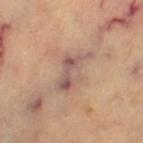{
  "biopsy_status": "not biopsied; imaged during a skin examination",
  "patient": {
    "sex": "female",
    "age_approx": 70
  },
  "lighting": "cross-polarized",
  "automated_metrics": {
    "area_mm2_approx": 10.0,
    "eccentricity": 0.85,
    "color_variation_0_10": 7.0,
    "peripheral_color_asymmetry": 2.0,
    "nevus_likeness_0_100": 0,
    "lesion_detection_confidence_0_100": 60
  },
  "image": {
    "source": "total-body photography crop",
    "field_of_view_mm": 15
  },
  "site": "left thigh"
}